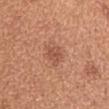From the chest. A male subject, aged 63 to 67. Imaged with white-light lighting. A close-up tile cropped from a whole-body skin photograph, about 15 mm across. About 3 mm across.